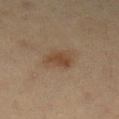Imaged with cross-polarized lighting. Longest diameter approximately 2.5 mm. Located on the leg. A female patient, roughly 50 years of age. Automated image analysis of the tile measured a lesion area of about 4.5 mm² and two-axis asymmetry of about 0.3. The analysis additionally found a border-irregularity index near 3/10, a color-variation rating of about 1.5/10, and radial color variation of about 0.5. The analysis additionally found an automated nevus-likeness rating near 90 out of 100. A 15 mm close-up extracted from a 3D total-body photography capture.